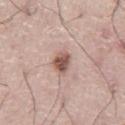Clinical impression:
Captured during whole-body skin photography for melanoma surveillance; the lesion was not biopsied.
Clinical summary:
A male subject, aged 73–77. A 15 mm crop from a total-body photograph taken for skin-cancer surveillance. This is a white-light tile. The recorded lesion diameter is about 3 mm. From the abdomen.